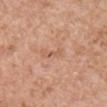Imaged during a routine full-body skin examination; the lesion was not biopsied and no histopathology is available. A lesion tile, about 15 mm wide, cut from a 3D total-body photograph. Captured under white-light illumination. Located on the right upper arm. The lesion-visualizer software estimated a footprint of about 2 mm² and an outline eccentricity of about 0.95 (0 = round, 1 = elongated). It also reported roughly 7 lightness units darker than nearby skin and a normalized lesion–skin contrast near 5. The software also gave a border-irregularity rating of about 3.5/10, a within-lesion color-variation index near 0/10, and a peripheral color-asymmetry measure near 0. It also reported a nevus-likeness score of about 0/100. A male patient, aged around 65. The recorded lesion diameter is about 2.5 mm.